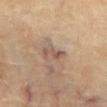Impression:
Imaged during a routine full-body skin examination; the lesion was not biopsied and no histopathology is available.
Clinical summary:
From the abdomen. This is a cross-polarized tile. A male patient, in their mid-70s. Measured at roughly 3.5 mm in maximum diameter. A roughly 15 mm field-of-view crop from a total-body skin photograph.